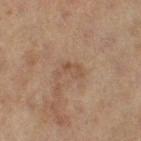  patient:
    sex: female
    age_approx: 60
  site: right thigh
  lighting: cross-polarized
  automated_metrics:
    cielab_L: 44
    cielab_a: 15
    cielab_b: 26
    vs_skin_darker_L: 6.0
    vs_skin_contrast_norm: 5.0
  image:
    source: total-body photography crop
    field_of_view_mm: 15
  lesion_size:
    long_diameter_mm_approx: 3.0